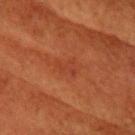biopsy status: no biopsy performed (imaged during a skin exam); lesion diameter: ~2.5 mm (longest diameter); body site: the head or neck; image source: ~15 mm tile from a whole-body skin photo; lighting: cross-polarized; patient: female, roughly 80 years of age.